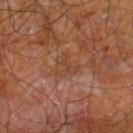The lesion was tiled from a total-body skin photograph and was not biopsied.
The subject is a male aged 58–62.
Measured at roughly 3 mm in maximum diameter.
Automated tile analysis of the lesion measured a lesion area of about 5 mm² and a shape-asymmetry score of about 0.55 (0 = symmetric). The software also gave a lesion color around L≈41 a*≈22 b*≈31 in CIELAB, a lesion–skin lightness drop of about 4, and a normalized lesion–skin contrast near 4.5.
A 15 mm close-up tile from a total-body photography series done for melanoma screening.
On the right leg.